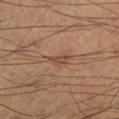No biopsy was performed on this lesion — it was imaged during a full skin examination and was not determined to be concerning. The patient is a male aged around 55. A close-up tile cropped from a whole-body skin photograph, about 15 mm across. The lesion is located on the right lower leg. Longest diameter approximately 2.5 mm. Automated tile analysis of the lesion measured a footprint of about 3.5 mm² and a symmetry-axis asymmetry near 0.35. It also reported about 7 CIELAB-L* units darker than the surrounding skin and a normalized lesion–skin contrast near 6. The software also gave a nevus-likeness score of about 5/100 and lesion-presence confidence of about 95/100.